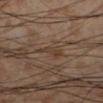Assessment: Imaged during a routine full-body skin examination; the lesion was not biopsied and no histopathology is available. Background: The lesion is located on the left lower leg. This is a cross-polarized tile. The recorded lesion diameter is about 3 mm. A lesion tile, about 15 mm wide, cut from a 3D total-body photograph. A male subject, in their mid- to late 50s.